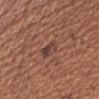{"image": {"source": "total-body photography crop", "field_of_view_mm": 15}, "patient": {"sex": "female", "age_approx": 50}, "lighting": "white-light", "automated_metrics": {"border_irregularity_0_10": 3.0, "color_variation_0_10": 7.0, "peripheral_color_asymmetry": 2.5, "nevus_likeness_0_100": 5, "lesion_detection_confidence_0_100": 95}, "lesion_size": {"long_diameter_mm_approx": 3.0}, "site": "right upper arm"}